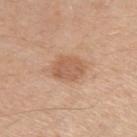The lesion was tiled from a total-body skin photograph and was not biopsied.
Measured at roughly 4 mm in maximum diameter.
A male patient aged around 60.
Automated image analysis of the tile measured a footprint of about 9 mm², an outline eccentricity of about 0.7 (0 = round, 1 = elongated), and a shape-asymmetry score of about 0.15 (0 = symmetric). The software also gave a border-irregularity index near 1.5/10 and a within-lesion color-variation index near 2.5/10. And it measured a lesion-detection confidence of about 100/100.
The lesion is located on the left upper arm.
Cropped from a total-body skin-imaging series; the visible field is about 15 mm.